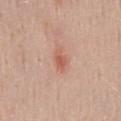workup: catalogued during a skin exam; not biopsied
tile lighting: white-light illumination
anatomic site: the mid back
subject: male, aged 38–42
image source: 15 mm crop, total-body photography
automated lesion analysis: an outline eccentricity of about 0.75 (0 = round, 1 = elongated) and two-axis asymmetry of about 0.3; a within-lesion color-variation index near 2.5/10; lesion-presence confidence of about 100/100